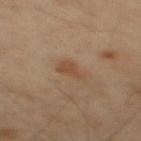Notes:
• notes · catalogued during a skin exam; not biopsied
• imaging modality · ~15 mm tile from a whole-body skin photo
• tile lighting · cross-polarized
• body site · the mid back
• patient · male, aged 38 to 42